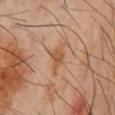{"biopsy_status": "not biopsied; imaged during a skin examination", "image": {"source": "total-body photography crop", "field_of_view_mm": 15}, "lighting": "cross-polarized", "site": "chest", "automated_metrics": {"area_mm2_approx": 4.5, "eccentricity": 0.75, "shape_asymmetry": 0.45, "vs_skin_darker_L": 8.0, "nevus_likeness_0_100": 45, "lesion_detection_confidence_0_100": 100}, "patient": {"sex": "male", "age_approx": 60}, "lesion_size": {"long_diameter_mm_approx": 3.0}}